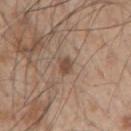This lesion was catalogued during total-body skin photography and was not selected for biopsy. A male subject, aged approximately 50. A close-up tile cropped from a whole-body skin photograph, about 15 mm across. The lesion is located on the right upper arm.